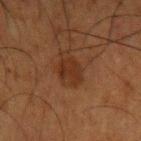Clinical impression: No biopsy was performed on this lesion — it was imaged during a full skin examination and was not determined to be concerning.